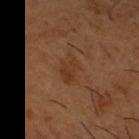Impression:
The lesion was photographed on a routine skin check and not biopsied; there is no pathology result.
Background:
Automated tile analysis of the lesion measured a lesion area of about 5 mm², an outline eccentricity of about 0.8 (0 = round, 1 = elongated), and two-axis asymmetry of about 0.25. The software also gave a lesion color around L≈35 a*≈21 b*≈31 in CIELAB. On the leg. The lesion's longest dimension is about 3 mm. This is a cross-polarized tile. A male patient aged around 65. This image is a 15 mm lesion crop taken from a total-body photograph.